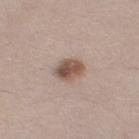• patient · female, aged approximately 40
• anatomic site · the right thigh
• tile lighting · white-light
• imaging modality · ~15 mm crop, total-body skin-cancer survey
• lesion diameter · ~3.5 mm (longest diameter)